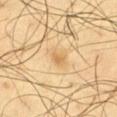  biopsy_status: not biopsied; imaged during a skin examination
  site: left thigh
  lighting: cross-polarized
  patient:
    sex: male
    age_approx: 65
  automated_metrics:
    area_mm2_approx: 2.5
    eccentricity: 0.45
    vs_skin_darker_L: 8.0
    vs_skin_contrast_norm: 6.0
    nevus_likeness_0_100: 0
    lesion_detection_confidence_0_100: 100
  lesion_size:
    long_diameter_mm_approx: 1.5
  image:
    source: total-body photography crop
    field_of_view_mm: 15